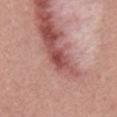The lesion was photographed on a routine skin check and not biopsied; there is no pathology result.